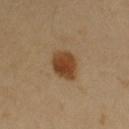size = ~4 mm (longest diameter) | image source = 15 mm crop, total-body photography | tile lighting = cross-polarized | patient = female, approximately 40 years of age | location = the right upper arm.